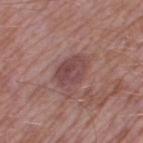Case summary:
- notes: total-body-photography surveillance lesion; no biopsy
- subject: male, in their mid- to late 50s
- TBP lesion metrics: an outline eccentricity of about 0.75 (0 = round, 1 = elongated) and a shape-asymmetry score of about 0.15 (0 = symmetric); an average lesion color of about L≈45 a*≈22 b*≈19 (CIELAB) and a lesion-to-skin contrast of about 7.5 (normalized; higher = more distinct); a within-lesion color-variation index near 4/10 and peripheral color asymmetry of about 1.5; a classifier nevus-likeness of about 15/100 and a lesion-detection confidence of about 100/100
- size: ≈3.5 mm
- imaging modality: 15 mm crop, total-body photography
- site: the right thigh